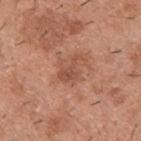Part of a total-body skin-imaging series; this lesion was reviewed on a skin check and was not flagged for biopsy.
A male patient in their 40s.
Approximately 3.5 mm at its widest.
A lesion tile, about 15 mm wide, cut from a 3D total-body photograph.
The total-body-photography lesion software estimated an outline eccentricity of about 0.85 (0 = round, 1 = elongated) and a shape-asymmetry score of about 0.6 (0 = symmetric). The software also gave a lesion color around L≈51 a*≈25 b*≈31 in CIELAB, about 8 CIELAB-L* units darker than the surrounding skin, and a normalized border contrast of about 6. The software also gave an automated nevus-likeness rating near 0 out of 100 and a detector confidence of about 100 out of 100 that the crop contains a lesion.
Located on the upper back.
This is a white-light tile.